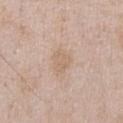No biopsy was performed on this lesion — it was imaged during a full skin examination and was not determined to be concerning.
The tile uses white-light illumination.
Longest diameter approximately 3 mm.
A male patient aged 48–52.
A roughly 15 mm field-of-view crop from a total-body skin photograph.
Automated image analysis of the tile measured a nevus-likeness score of about 0/100 and a lesion-detection confidence of about 100/100.
The lesion is located on the chest.